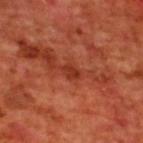This lesion was catalogued during total-body skin photography and was not selected for biopsy.
The lesion is on the upper back.
A 15 mm close-up tile from a total-body photography series done for melanoma screening.
The lesion's longest dimension is about 2.5 mm.
The subject is a male about 70 years old.